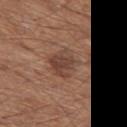follow-up=imaged on a skin check; not biopsied | subject=male, aged around 65 | size=~3.5 mm (longest diameter) | acquisition=~15 mm tile from a whole-body skin photo | anatomic site=the left thigh | illumination=white-light.